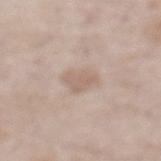<lesion>
  <biopsy_status>not biopsied; imaged during a skin examination</biopsy_status>
  <site>front of the torso</site>
  <patient>
    <sex>male</sex>
    <age_approx>55</age_approx>
  </patient>
  <lighting>white-light</lighting>
  <image>
    <source>total-body photography crop</source>
    <field_of_view_mm>15</field_of_view_mm>
  </image>
  <lesion_size>
    <long_diameter_mm_approx>3.5</long_diameter_mm_approx>
  </lesion_size>
  <automated_metrics>
    <area_mm2_approx>7.0</area_mm2_approx>
    <eccentricity>0.55</eccentricity>
    <cielab_L>63</cielab_L>
    <cielab_a>14</cielab_a>
    <cielab_b>25</cielab_b>
    <vs_skin_darker_L>7.0</vs_skin_darker_L>
    <vs_skin_contrast_norm>5.0</vs_skin_contrast_norm>
    <lesion_detection_confidence_0_100>100</lesion_detection_confidence_0_100>
  </automated_metrics>
</lesion>